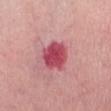Notes:
• body site — the mid back
• patient — female, aged approximately 65
• lighting — white-light illumination
• image — 15 mm crop, total-body photography